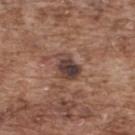The lesion was photographed on a routine skin check and not biopsied; there is no pathology result. The lesion's longest dimension is about 3.5 mm. A male subject roughly 75 years of age. A close-up tile cropped from a whole-body skin photograph, about 15 mm across. The lesion is on the upper back.